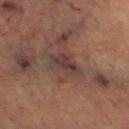Q: Was this lesion biopsied?
A: imaged on a skin check; not biopsied
Q: What is the anatomic site?
A: the leg
Q: How was this image acquired?
A: 15 mm crop, total-body photography
Q: What are the patient's age and sex?
A: female, in their mid- to late 60s
Q: Lesion size?
A: ≈4 mm
Q: What lighting was used for the tile?
A: cross-polarized
Q: What did automated image analysis measure?
A: a symmetry-axis asymmetry near 0.3; a border-irregularity index near 3.5/10 and a within-lesion color-variation index near 3.5/10; a detector confidence of about 55 out of 100 that the crop contains a lesion